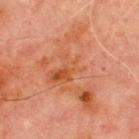The lesion was tiled from a total-body skin photograph and was not biopsied.
The lesion is located on the chest.
A region of skin cropped from a whole-body photographic capture, roughly 15 mm wide.
Automated image analysis of the tile measured a lesion area of about 31 mm², a shape eccentricity near 0.8, and two-axis asymmetry of about 0.65. It also reported a mean CIELAB color near L≈46 a*≈24 b*≈32, about 6 CIELAB-L* units darker than the surrounding skin, and a normalized lesion–skin contrast near 6. The analysis additionally found a border-irregularity index near 10/10 and a within-lesion color-variation index near 8/10.
A male patient aged approximately 70.
This is a cross-polarized tile.